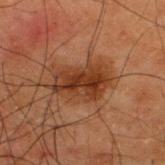Assessment:
Part of a total-body skin-imaging series; this lesion was reviewed on a skin check and was not flagged for biopsy.
Clinical summary:
The recorded lesion diameter is about 5.5 mm. A male patient roughly 50 years of age. A region of skin cropped from a whole-body photographic capture, roughly 15 mm wide. Located on the upper back. This is a cross-polarized tile.